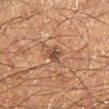Impression:
Part of a total-body skin-imaging series; this lesion was reviewed on a skin check and was not flagged for biopsy.
Image and clinical context:
The patient is a male aged approximately 60. On the right lower leg. A region of skin cropped from a whole-body photographic capture, roughly 15 mm wide. Longest diameter approximately 2.5 mm. Imaged with cross-polarized lighting.